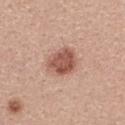Notes:
– notes — imaged on a skin check; not biopsied
– size — ≈4 mm
– patient — female, aged around 40
– imaging modality — ~15 mm tile from a whole-body skin photo
– tile lighting — white-light illumination
– anatomic site — the upper back